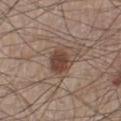The lesion was photographed on a routine skin check and not biopsied; there is no pathology result. The recorded lesion diameter is about 3.5 mm. The subject is a male aged 58–62. Located on the left lower leg. A 15 mm crop from a total-body photograph taken for skin-cancer surveillance. Imaged with white-light lighting. The lesion-visualizer software estimated border irregularity of about 2.5 on a 0–10 scale, internal color variation of about 4 on a 0–10 scale, and peripheral color asymmetry of about 1.